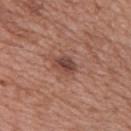{
  "biopsy_status": "not biopsied; imaged during a skin examination",
  "site": "upper back",
  "image": {
    "source": "total-body photography crop",
    "field_of_view_mm": 15
  },
  "automated_metrics": {
    "area_mm2_approx": 4.5,
    "eccentricity": 0.7,
    "cielab_L": 44,
    "cielab_a": 22,
    "cielab_b": 24,
    "vs_skin_contrast_norm": 8.5
  },
  "lesion_size": {
    "long_diameter_mm_approx": 3.0
  },
  "patient": {
    "sex": "female",
    "age_approx": 40
  },
  "lighting": "white-light"
}